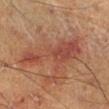  biopsy_status: not biopsied; imaged during a skin examination